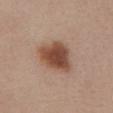The lesion was photographed on a routine skin check and not biopsied; there is no pathology result. Measured at roughly 5 mm in maximum diameter. The tile uses white-light illumination. On the abdomen. The subject is a female in their mid-50s. A lesion tile, about 15 mm wide, cut from a 3D total-body photograph. The lesion-visualizer software estimated a footprint of about 14 mm² and two-axis asymmetry of about 0.25. The analysis additionally found a lesion color around L≈48 a*≈21 b*≈29 in CIELAB, roughly 14 lightness units darker than nearby skin, and a normalized lesion–skin contrast near 10.5. And it measured a border-irregularity rating of about 2.5/10, a within-lesion color-variation index near 4/10, and peripheral color asymmetry of about 1.